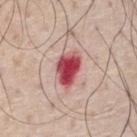follow-up: total-body-photography surveillance lesion; no biopsy | image source: 15 mm crop, total-body photography | patient: male, aged approximately 70 | tile lighting: white-light illumination | TBP lesion metrics: a lesion color around L≈50 a*≈37 b*≈23 in CIELAB and a lesion-to-skin contrast of about 13.5 (normalized; higher = more distinct); an automated nevus-likeness rating near 0 out of 100 and a detector confidence of about 100 out of 100 that the crop contains a lesion | anatomic site: the front of the torso.